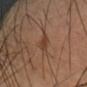Impression: No biopsy was performed on this lesion — it was imaged during a full skin examination and was not determined to be concerning. Acquisition and patient details: The lesion is located on the arm. The subject is a male in their mid- to late 40s. A lesion tile, about 15 mm wide, cut from a 3D total-body photograph.